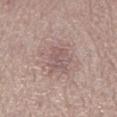Q: Is there a histopathology result?
A: catalogued during a skin exam; not biopsied
Q: What are the patient's age and sex?
A: female, aged 48–52
Q: What is the lesion's diameter?
A: ~3 mm (longest diameter)
Q: What is the imaging modality?
A: total-body-photography crop, ~15 mm field of view
Q: What is the anatomic site?
A: the left lower leg
Q: How was the tile lit?
A: white-light